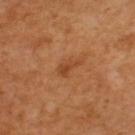{
  "site": "left upper arm",
  "image": {
    "source": "total-body photography crop",
    "field_of_view_mm": 15
  },
  "lighting": "cross-polarized",
  "patient": {
    "sex": "female",
    "age_approx": 55
  }
}